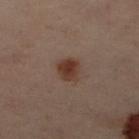Clinical impression: No biopsy was performed on this lesion — it was imaged during a full skin examination and was not determined to be concerning. Image and clinical context: This is a cross-polarized tile. The patient is a female in their 60s. The lesion is on the left leg. A lesion tile, about 15 mm wide, cut from a 3D total-body photograph. About 3 mm across.